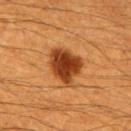Imaged during a routine full-body skin examination; the lesion was not biopsied and no histopathology is available. This is a cross-polarized tile. The subject is a male in their 60s. Located on the upper back. Approximately 4.5 mm at its widest. A close-up tile cropped from a whole-body skin photograph, about 15 mm across.